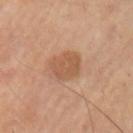Part of a total-body skin-imaging series; this lesion was reviewed on a skin check and was not flagged for biopsy.
The lesion's longest dimension is about 4 mm.
From the arm.
A male patient roughly 60 years of age.
Imaged with cross-polarized lighting.
Cropped from a total-body skin-imaging series; the visible field is about 15 mm.